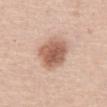<record>
<biopsy_status>not biopsied; imaged during a skin examination</biopsy_status>
<lighting>white-light</lighting>
<image>
  <source>total-body photography crop</source>
  <field_of_view_mm>15</field_of_view_mm>
</image>
<patient>
  <sex>male</sex>
  <age_approx>50</age_approx>
</patient>
<site>abdomen</site>
<lesion_size>
  <long_diameter_mm_approx>5.0</long_diameter_mm_approx>
</lesion_size>
</record>